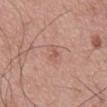| feature | finding |
|---|---|
| follow-up | imaged on a skin check; not biopsied |
| lighting | white-light |
| patient | male, aged approximately 55 |
| image source | ~15 mm tile from a whole-body skin photo |
| site | the back |
| lesion diameter | ~3 mm (longest diameter) |